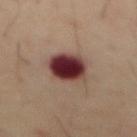Clinical impression:
Captured during whole-body skin photography for melanoma surveillance; the lesion was not biopsied.
Acquisition and patient details:
Imaged with cross-polarized lighting. The lesion is located on the abdomen. A male patient, in their 70s. Longest diameter approximately 4.5 mm. This image is a 15 mm lesion crop taken from a total-body photograph.